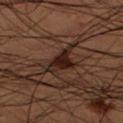biopsy status: catalogued during a skin exam; not biopsied
image-analysis metrics: an area of roughly 6.5 mm², an outline eccentricity of about 0.85 (0 = round, 1 = elongated), and two-axis asymmetry of about 0.5; a border-irregularity index near 5/10 and a peripheral color-asymmetry measure near 0.5; a classifier nevus-likeness of about 100/100
body site: the left lower leg
subject: male, approximately 50 years of age
image source: 15 mm crop, total-body photography